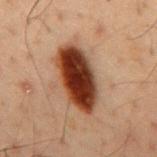A male patient approximately 50 years of age. Longest diameter approximately 7.5 mm. From the mid back. Imaged with cross-polarized lighting. Cropped from a total-body skin-imaging series; the visible field is about 15 mm. The total-body-photography lesion software estimated a footprint of about 20 mm², an eccentricity of roughly 0.9, and two-axis asymmetry of about 0.1. And it measured a mean CIELAB color near L≈28 a*≈20 b*≈25, about 19 CIELAB-L* units darker than the surrounding skin, and a lesion-to-skin contrast of about 16.5 (normalized; higher = more distinct). And it measured a border-irregularity rating of about 1.5/10, a color-variation rating of about 6.5/10, and radial color variation of about 2. And it measured a classifier nevus-likeness of about 100/100.